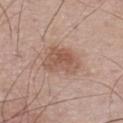follow-up = imaged on a skin check; not biopsied
automated metrics = a mean CIELAB color near L≈54 a*≈19 b*≈27 and a normalized lesion–skin contrast near 7; border irregularity of about 3 on a 0–10 scale, a color-variation rating of about 4/10, and peripheral color asymmetry of about 1.5; lesion-presence confidence of about 100/100
body site = the upper back
image source = ~15 mm tile from a whole-body skin photo
diameter = ~4 mm (longest diameter)
patient = male, in their mid- to late 40s
tile lighting = white-light illumination